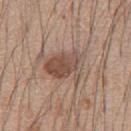This lesion was catalogued during total-body skin photography and was not selected for biopsy. A roughly 15 mm field-of-view crop from a total-body skin photograph. A male patient, roughly 60 years of age. From the chest.